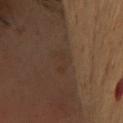notes=catalogued during a skin exam; not biopsied
image=15 mm crop, total-body photography
size=~3.5 mm (longest diameter)
image-analysis metrics=a footprint of about 5 mm², an eccentricity of roughly 0.8, and a symmetry-axis asymmetry near 0.4; a border-irregularity rating of about 4.5/10, a color-variation rating of about 1.5/10, and radial color variation of about 0.5; an automated nevus-likeness rating near 0 out of 100
location=the head or neck
tile lighting=cross-polarized
patient=female, aged 58 to 62